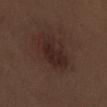imaging modality: 15 mm crop, total-body photography; patient: male, aged around 70; body site: the left upper arm; diameter: ≈4 mm; lighting: white-light illumination.